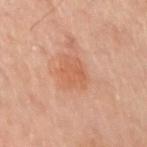| feature | finding |
|---|---|
| workup | no biopsy performed (imaged during a skin exam) |
| location | the left arm |
| patient | female, approximately 60 years of age |
| image | ~15 mm crop, total-body skin-cancer survey |
| lesion size | ~4 mm (longest diameter) |